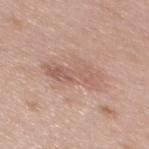Notes:
– size: about 6 mm
– image: ~15 mm crop, total-body skin-cancer survey
– patient: female, about 40 years old
– site: the mid back